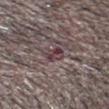follow-up: imaged on a skin check; not biopsied
subject: male, aged 38 to 42
location: the head or neck
lesion diameter: about 2.5 mm
image-analysis metrics: about 9 CIELAB-L* units darker than the surrounding skin and a normalized lesion–skin contrast near 8; border irregularity of about 2.5 on a 0–10 scale, a color-variation rating of about 6/10, and radial color variation of about 2
imaging modality: ~15 mm crop, total-body skin-cancer survey
tile lighting: white-light illumination